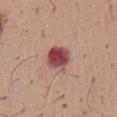Impression: Imaged during a routine full-body skin examination; the lesion was not biopsied and no histopathology is available. Context: A 15 mm close-up extracted from a 3D total-body photography capture. The lesion is located on the chest. The subject is a male aged approximately 60.